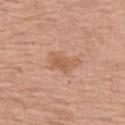biopsy_status: not biopsied; imaged during a skin examination
image:
  source: total-body photography crop
  field_of_view_mm: 15
automated_metrics:
  area_mm2_approx: 6.5
  eccentricity: 0.85
  cielab_L: 58
  cielab_a: 22
  cielab_b: 33
  vs_skin_darker_L: 8.0
  vs_skin_contrast_norm: 5.5
  border_irregularity_0_10: 4.0
  color_variation_0_10: 2.5
  peripheral_color_asymmetry: 0.5
  lesion_detection_confidence_0_100: 100
site: left thigh
lighting: white-light
patient:
  sex: female
  age_approx: 65
lesion_size:
  long_diameter_mm_approx: 4.0